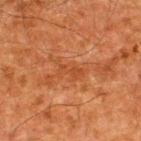Captured during whole-body skin photography for melanoma surveillance; the lesion was not biopsied.
Located on the upper back.
A 15 mm crop from a total-body photograph taken for skin-cancer surveillance.
Captured under cross-polarized illumination.
The patient is a male aged around 60.